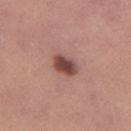Captured during whole-body skin photography for melanoma surveillance; the lesion was not biopsied. A region of skin cropped from a whole-body photographic capture, roughly 15 mm wide. The subject is a female aged 23–27. The lesion is located on the leg. The lesion's longest dimension is about 3 mm. The total-body-photography lesion software estimated a footprint of about 5.5 mm² and an eccentricity of roughly 0.75. The analysis additionally found a lesion color around L≈46 a*≈23 b*≈22 in CIELAB and a lesion-to-skin contrast of about 10.5 (normalized; higher = more distinct). The tile uses white-light illumination.